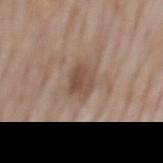| field | value |
|---|---|
| biopsy status | total-body-photography surveillance lesion; no biopsy |
| lighting | white-light illumination |
| subject | male, about 60 years old |
| body site | the mid back |
| image | ~15 mm crop, total-body skin-cancer survey |
| diameter | ≈3 mm |